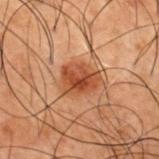Q: Lesion location?
A: the upper back
Q: Who is the patient?
A: male, in their 50s
Q: How was this image acquired?
A: total-body-photography crop, ~15 mm field of view
Q: Automated lesion metrics?
A: border irregularity of about 2 on a 0–10 scale, a color-variation rating of about 5/10, and a peripheral color-asymmetry measure near 2; a nevus-likeness score of about 95/100